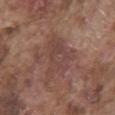| field | value |
|---|---|
| workup | no biopsy performed (imaged during a skin exam) |
| anatomic site | the front of the torso |
| size | ≈6 mm |
| tile lighting | white-light illumination |
| subject | male, roughly 75 years of age |
| image source | 15 mm crop, total-body photography |